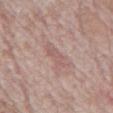Captured during whole-body skin photography for melanoma surveillance; the lesion was not biopsied.
On the abdomen.
The subject is a male approximately 70 years of age.
A region of skin cropped from a whole-body photographic capture, roughly 15 mm wide.
The tile uses white-light illumination.
Longest diameter approximately 3.5 mm.